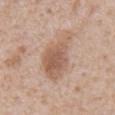From the chest.
The lesion's longest dimension is about 7 mm.
Automated image analysis of the tile measured an average lesion color of about L≈58 a*≈18 b*≈29 (CIELAB), roughly 10 lightness units darker than nearby skin, and a normalized border contrast of about 7. The software also gave a border-irregularity rating of about 4.5/10, a color-variation rating of about 4/10, and a peripheral color-asymmetry measure near 1. The software also gave lesion-presence confidence of about 100/100.
A 15 mm close-up tile from a total-body photography series done for melanoma screening.
The tile uses white-light illumination.
A male patient aged around 65.